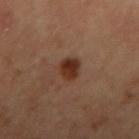{"biopsy_status": "not biopsied; imaged during a skin examination", "automated_metrics": {"eccentricity": 0.55, "shape_asymmetry": 0.2, "border_irregularity_0_10": 1.5, "color_variation_0_10": 4.0, "peripheral_color_asymmetry": 1.5}, "image": {"source": "total-body photography crop", "field_of_view_mm": 15}, "patient": {"sex": "female", "age_approx": 45}, "lesion_size": {"long_diameter_mm_approx": 3.0}, "site": "right upper arm"}